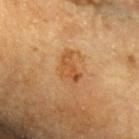Recorded during total-body skin imaging; not selected for excision or biopsy. The tile uses cross-polarized illumination. The lesion's longest dimension is about 4 mm. From the head or neck. A male patient in their mid- to late 80s or older. A 15 mm close-up extracted from a 3D total-body photography capture.